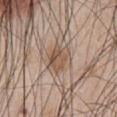| field | value |
|---|---|
| follow-up | imaged on a skin check; not biopsied |
| subject | male, aged 43–47 |
| image | 15 mm crop, total-body photography |
| body site | the abdomen |
| illumination | white-light illumination |
| lesion diameter | about 3.5 mm |